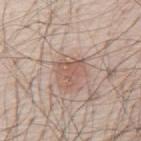notes=catalogued during a skin exam; not biopsied
patient=male, aged 78–82
image source=~15 mm crop, total-body skin-cancer survey
site=the mid back